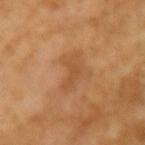{
  "biopsy_status": "not biopsied; imaged during a skin examination",
  "site": "right forearm",
  "image": {
    "source": "total-body photography crop",
    "field_of_view_mm": 15
  },
  "patient": {
    "sex": "female",
    "age_approx": 70
  },
  "lesion_size": {
    "long_diameter_mm_approx": 3.5
  }
}